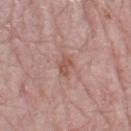Assessment:
The lesion was tiled from a total-body skin photograph and was not biopsied.
Image and clinical context:
The subject is a female in their mid- to late 60s. A lesion tile, about 15 mm wide, cut from a 3D total-body photograph. From the left thigh. Captured under white-light illumination. The lesion's longest dimension is about 2.5 mm.